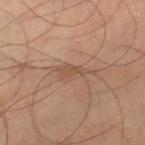Notes:
• diameter · about 4.5 mm
• location · the right thigh
• automated lesion analysis · a shape eccentricity near 0.9 and a symmetry-axis asymmetry near 0.45
• illumination · cross-polarized
• subject · male, aged approximately 60
• image source · 15 mm crop, total-body photography